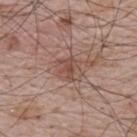A lesion tile, about 15 mm wide, cut from a 3D total-body photograph. Captured under white-light illumination. From the upper back. A male patient aged 63–67. Approximately 3 mm at its widest. The total-body-photography lesion software estimated a footprint of about 5.5 mm², an outline eccentricity of about 0.45 (0 = round, 1 = elongated), and a shape-asymmetry score of about 0.35 (0 = symmetric). The analysis additionally found about 8 CIELAB-L* units darker than the surrounding skin and a normalized lesion–skin contrast near 6. The analysis additionally found a border-irregularity index near 4/10, internal color variation of about 2.5 on a 0–10 scale, and radial color variation of about 1. The software also gave a nevus-likeness score of about 0/100 and lesion-presence confidence of about 100/100.